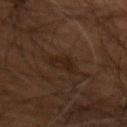No biopsy was performed on this lesion — it was imaged during a full skin examination and was not determined to be concerning.
Cropped from a total-body skin-imaging series; the visible field is about 15 mm.
A male patient roughly 55 years of age.
Imaged with cross-polarized lighting.
About 3.5 mm across.
Automated tile analysis of the lesion measured an area of roughly 5.5 mm², a shape eccentricity near 0.75, and two-axis asymmetry of about 0.4. And it measured a nevus-likeness score of about 0/100.
On the arm.